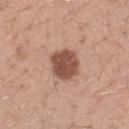Impression: This lesion was catalogued during total-body skin photography and was not selected for biopsy. Background: Measured at roughly 3.5 mm in maximum diameter. A male patient aged around 30. A close-up tile cropped from a whole-body skin photograph, about 15 mm across. On the left forearm. Imaged with white-light lighting.